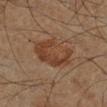Cropped from a total-body skin-imaging series; the visible field is about 15 mm. This is a cross-polarized tile. A male patient in their mid-60s. The lesion is on the left lower leg.